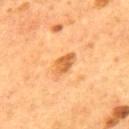Clinical impression: This lesion was catalogued during total-body skin photography and was not selected for biopsy. Image and clinical context: A male patient, about 55 years old. The lesion is on the mid back. Approximately 3.5 mm at its widest. This is a cross-polarized tile. Cropped from a whole-body photographic skin survey; the tile spans about 15 mm. The total-body-photography lesion software estimated a lesion area of about 5.5 mm². And it measured a lesion color around L≈59 a*≈26 b*≈45 in CIELAB and about 11 CIELAB-L* units darker than the surrounding skin.